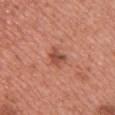No biopsy was performed on this lesion — it was imaged during a full skin examination and was not determined to be concerning. A region of skin cropped from a whole-body photographic capture, roughly 15 mm wide. The lesion is located on the front of the torso. The subject is a female aged approximately 40. The lesion's longest dimension is about 2.5 mm. Captured under white-light illumination.